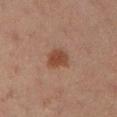Recorded during total-body skin imaging; not selected for excision or biopsy.
A 15 mm close-up extracted from a 3D total-body photography capture.
The lesion is located on the left lower leg.
Imaged with cross-polarized lighting.
A female subject, aged 18 to 22.